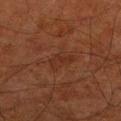Assessment:
Imaged during a routine full-body skin examination; the lesion was not biopsied and no histopathology is available.
Context:
From the leg. The patient is a male in their 80s. Imaged with cross-polarized lighting. About 3 mm across. Cropped from a total-body skin-imaging series; the visible field is about 15 mm.